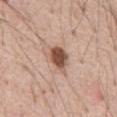biopsy status: catalogued during a skin exam; not biopsied | image: ~15 mm tile from a whole-body skin photo | subject: male, aged around 55 | TBP lesion metrics: a footprint of about 7 mm², a shape eccentricity near 0.7, and two-axis asymmetry of about 0.25; a mean CIELAB color near L≈52 a*≈20 b*≈28, roughly 16 lightness units darker than nearby skin, and a normalized lesion–skin contrast near 11; a border-irregularity index near 2.5/10, a within-lesion color-variation index near 4/10, and a peripheral color-asymmetry measure near 1; an automated nevus-likeness rating near 95 out of 100 and lesion-presence confidence of about 100/100 | body site: the abdomen.